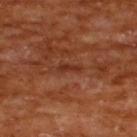| field | value |
|---|---|
| biopsy status | imaged on a skin check; not biopsied |
| imaging modality | ~15 mm tile from a whole-body skin photo |
| subject | male, roughly 65 years of age |
| diameter | about 3 mm |
| location | the upper back |
| illumination | cross-polarized |